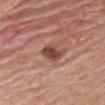biopsy status: catalogued during a skin exam; not biopsied | TBP lesion metrics: a footprint of about 6 mm², an eccentricity of roughly 0.8, and two-axis asymmetry of about 0.3; an average lesion color of about L≈46 a*≈24 b*≈27 (CIELAB) and a lesion-to-skin contrast of about 9 (normalized; higher = more distinct); an automated nevus-likeness rating near 55 out of 100 and lesion-presence confidence of about 100/100 | subject: male, aged 83 or older | size: ≈3.5 mm | body site: the chest | lighting: white-light illumination | imaging modality: ~15 mm crop, total-body skin-cancer survey.